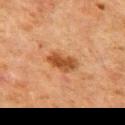Q: Was this lesion biopsied?
A: total-body-photography surveillance lesion; no biopsy